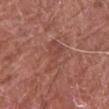Recorded during total-body skin imaging; not selected for excision or biopsy. The tile uses white-light illumination. A male patient, approximately 80 years of age. A 15 mm close-up tile from a total-body photography series done for melanoma screening. Located on the arm.